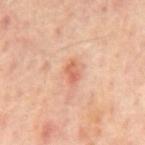notes: imaged on a skin check; not biopsied
lighting: cross-polarized illumination
imaging modality: 15 mm crop, total-body photography
size: about 2.5 mm
automated metrics: an area of roughly 3.5 mm² and a shape-asymmetry score of about 0.3 (0 = symmetric); an average lesion color of about L≈61 a*≈26 b*≈33 (CIELAB) and a lesion-to-skin contrast of about 6.5 (normalized; higher = more distinct); internal color variation of about 3 on a 0–10 scale and peripheral color asymmetry of about 1; a classifier nevus-likeness of about 25/100 and lesion-presence confidence of about 100/100
patient: male, aged approximately 65
anatomic site: the back